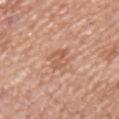<record>
  <biopsy_status>not biopsied; imaged during a skin examination</biopsy_status>
  <patient>
    <sex>male</sex>
    <age_approx>75</age_approx>
  </patient>
  <image>
    <source>total-body photography crop</source>
    <field_of_view_mm>15</field_of_view_mm>
  </image>
  <site>chest</site>
  <lighting>white-light</lighting>
</record>